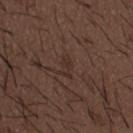| key | value |
|---|---|
| subject | male, aged 48–52 |
| size | ≈2.5 mm |
| anatomic site | the mid back |
| tile lighting | white-light |
| image-analysis metrics | a mean CIELAB color near L≈29 a*≈16 b*≈21, about 5 CIELAB-L* units darker than the surrounding skin, and a lesion-to-skin contrast of about 5 (normalized; higher = more distinct); a lesion-detection confidence of about 95/100 |
| image source | ~15 mm crop, total-body skin-cancer survey |